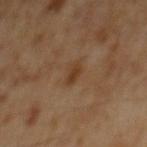Imaged during a routine full-body skin examination; the lesion was not biopsied and no histopathology is available. Measured at roughly 2.5 mm in maximum diameter. Cropped from a total-body skin-imaging series; the visible field is about 15 mm. The subject is a male aged 58 to 62. Located on the mid back. The total-body-photography lesion software estimated an area of roughly 3 mm² and a symmetry-axis asymmetry near 0.3. And it measured a mean CIELAB color near L≈34 a*≈17 b*≈29, about 7 CIELAB-L* units darker than the surrounding skin, and a lesion-to-skin contrast of about 7 (normalized; higher = more distinct). It also reported a classifier nevus-likeness of about 20/100 and a lesion-detection confidence of about 100/100.